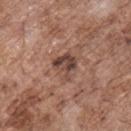workup=catalogued during a skin exam; not biopsied | lighting=white-light | automated lesion analysis=a lesion area of about 6.5 mm², a shape eccentricity near 0.45, and two-axis asymmetry of about 0.4; a normalized border contrast of about 9 | site=the chest | diameter=~3 mm (longest diameter) | subject=male, in their 70s | imaging modality=15 mm crop, total-body photography.